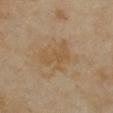Clinical impression: The lesion was photographed on a routine skin check and not biopsied; there is no pathology result. Context: Located on the chest. Cropped from a whole-body photographic skin survey; the tile spans about 15 mm. A female patient, about 35 years old. Automated tile analysis of the lesion measured an outline eccentricity of about 0.75 (0 = round, 1 = elongated) and two-axis asymmetry of about 0.4. And it measured a mean CIELAB color near L≈50 a*≈14 b*≈33, a lesion–skin lightness drop of about 5, and a normalized lesion–skin contrast near 5.5. It also reported a within-lesion color-variation index near 1.5/10 and radial color variation of about 0.5. The software also gave a lesion-detection confidence of about 100/100. Imaged with cross-polarized lighting.